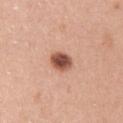This lesion was catalogued during total-body skin photography and was not selected for biopsy.